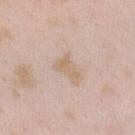| key | value |
|---|---|
| notes | catalogued during a skin exam; not biopsied |
| anatomic site | the left forearm |
| patient | female, approximately 25 years of age |
| illumination | white-light |
| acquisition | ~15 mm crop, total-body skin-cancer survey |